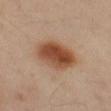A 15 mm crop from a total-body photograph taken for skin-cancer surveillance.
Measured at roughly 5.5 mm in maximum diameter.
Captured under cross-polarized illumination.
A female patient, aged 43–47.
The lesion is on the left thigh.